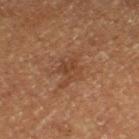<lesion>
  <biopsy_status>not biopsied; imaged during a skin examination</biopsy_status>
  <patient>
    <sex>male</sex>
    <age_approx>75</age_approx>
  </patient>
  <image>
    <source>total-body photography crop</source>
    <field_of_view_mm>15</field_of_view_mm>
  </image>
  <site>leg</site>
</lesion>